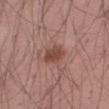workup = catalogued during a skin exam; not biopsied | size = ≈3 mm | anatomic site = the right thigh | image = total-body-photography crop, ~15 mm field of view | patient = male, aged around 55.